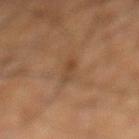Imaged during a routine full-body skin examination; the lesion was not biopsied and no histopathology is available.